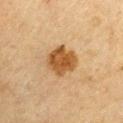Context: Approximately 4 mm at its widest. Automated image analysis of the tile measured a lesion area of about 11 mm², an outline eccentricity of about 0.4 (0 = round, 1 = elongated), and a shape-asymmetry score of about 0.1 (0 = symmetric). The analysis additionally found an average lesion color of about L≈42 a*≈18 b*≈35 (CIELAB), roughly 12 lightness units darker than nearby skin, and a lesion-to-skin contrast of about 10 (normalized; higher = more distinct). Located on the left upper arm. A 15 mm crop from a total-body photograph taken for skin-cancer surveillance. This is a cross-polarized tile. A male subject, about 65 years old.